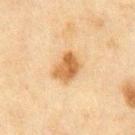Q: Was a biopsy performed?
A: no biopsy performed (imaged during a skin exam)
Q: Automated lesion metrics?
A: a footprint of about 8.5 mm², an outline eccentricity of about 0.7 (0 = round, 1 = elongated), and two-axis asymmetry of about 0.25; an automated nevus-likeness rating near 100 out of 100 and a detector confidence of about 100 out of 100 that the crop contains a lesion
Q: What is the lesion's diameter?
A: ≈4 mm
Q: Lesion location?
A: the chest
Q: What kind of image is this?
A: total-body-photography crop, ~15 mm field of view
Q: Patient demographics?
A: male, about 70 years old
Q: What lighting was used for the tile?
A: cross-polarized